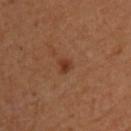{"biopsy_status": "not biopsied; imaged during a skin examination", "site": "upper back", "lesion_size": {"long_diameter_mm_approx": 2.0}, "automated_metrics": {"area_mm2_approx": 2.5, "eccentricity": 0.5, "shape_asymmetry": 0.3, "border_irregularity_0_10": 3.0, "color_variation_0_10": 2.5, "peripheral_color_asymmetry": 1.0}, "image": {"source": "total-body photography crop", "field_of_view_mm": 15}, "patient": {"sex": "male", "age_approx": 65}}